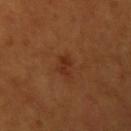workup: no biopsy performed (imaged during a skin exam)
acquisition: ~15 mm crop, total-body skin-cancer survey
site: the left upper arm
subject: female, aged 53–57